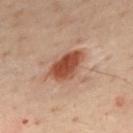Impression:
The lesion was tiled from a total-body skin photograph and was not biopsied.
Background:
A male patient aged 48 to 52. The recorded lesion diameter is about 4.5 mm. A close-up tile cropped from a whole-body skin photograph, about 15 mm across. The lesion is located on the mid back. Captured under cross-polarized illumination. The total-body-photography lesion software estimated a footprint of about 10 mm² and a shape-asymmetry score of about 0.2 (0 = symmetric). The software also gave a border-irregularity index near 2/10, internal color variation of about 3 on a 0–10 scale, and peripheral color asymmetry of about 1.